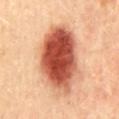<lesion>
<biopsy_status>not biopsied; imaged during a skin examination</biopsy_status>
<image>
  <source>total-body photography crop</source>
  <field_of_view_mm>15</field_of_view_mm>
</image>
<site>back</site>
<patient>
  <sex>female</sex>
  <age_approx>45</age_approx>
</patient>
</lesion>